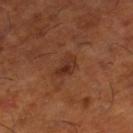workup: catalogued during a skin exam; not biopsied | body site: the leg | subject: male, roughly 65 years of age | acquisition: 15 mm crop, total-body photography | lesion diameter: ~3 mm (longest diameter).